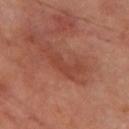notes = imaged on a skin check; not biopsied | patient = male, about 70 years old | body site = the left thigh | imaging modality = total-body-photography crop, ~15 mm field of view.